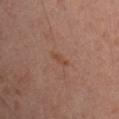follow-up — no biopsy performed (imaged during a skin exam) | image source — 15 mm crop, total-body photography | TBP lesion metrics — an outline eccentricity of about 0.95 (0 = round, 1 = elongated) and a shape-asymmetry score of about 0.45 (0 = symmetric); a mean CIELAB color near L≈43 a*≈21 b*≈28 and about 6 CIELAB-L* units darker than the surrounding skin; a color-variation rating of about 0/10 and peripheral color asymmetry of about 0; a lesion-detection confidence of about 100/100 | location — the left arm | patient — male, approximately 35 years of age | tile lighting — cross-polarized illumination | lesion diameter — ≈2.5 mm.